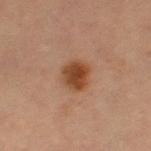Case summary:
* workup — no biopsy performed (imaged during a skin exam)
* location — the leg
* diameter — about 3.5 mm
* subject — female, roughly 70 years of age
* illumination — cross-polarized illumination
* image source — total-body-photography crop, ~15 mm field of view